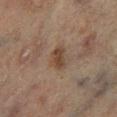No biopsy was performed on this lesion — it was imaged during a full skin examination and was not determined to be concerning. Longest diameter approximately 3 mm. Located on the left lower leg. A 15 mm close-up tile from a total-body photography series done for melanoma screening. Captured under cross-polarized illumination. A male subject about 70 years old.